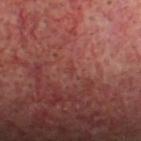{
  "biopsy_status": "not biopsied; imaged during a skin examination",
  "lesion_size": {
    "long_diameter_mm_approx": 1.0
  },
  "site": "head or neck",
  "patient": {
    "sex": "male",
    "age_approx": 50
  },
  "image": {
    "source": "total-body photography crop",
    "field_of_view_mm": 15
  },
  "automated_metrics": {
    "cielab_L": 39,
    "cielab_a": 28,
    "cielab_b": 23,
    "vs_skin_darker_L": 4.0
  },
  "lighting": "cross-polarized"
}